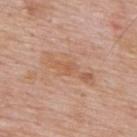<record>
  <biopsy_status>not biopsied; imaged during a skin examination</biopsy_status>
  <image>
    <source>total-body photography crop</source>
    <field_of_view_mm>15</field_of_view_mm>
  </image>
  <site>upper back</site>
  <automated_metrics>
    <border_irregularity_0_10>6.0</border_irregularity_0_10>
    <peripheral_color_asymmetry>0.5</peripheral_color_asymmetry>
    <nevus_likeness_0_100>0</nevus_likeness_0_100>
    <lesion_detection_confidence_0_100>100</lesion_detection_confidence_0_100>
  </automated_metrics>
  <patient>
    <sex>male</sex>
    <age_approx>60</age_approx>
  </patient>
  <lesion_size>
    <long_diameter_mm_approx>6.5</long_diameter_mm_approx>
  </lesion_size>
</record>